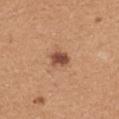Clinical impression: Imaged during a routine full-body skin examination; the lesion was not biopsied and no histopathology is available. Acquisition and patient details: A lesion tile, about 15 mm wide, cut from a 3D total-body photograph. An algorithmic analysis of the crop reported an area of roughly 4.5 mm², an eccentricity of roughly 0.7, and a shape-asymmetry score of about 0.15 (0 = symmetric). The analysis additionally found an average lesion color of about L≈49 a*≈23 b*≈31 (CIELAB), about 14 CIELAB-L* units darker than the surrounding skin, and a normalized border contrast of about 10. And it measured border irregularity of about 1 on a 0–10 scale, a within-lesion color-variation index near 2.5/10, and a peripheral color-asymmetry measure near 0.5. A female subject aged approximately 40. Imaged with white-light lighting. Located on the upper back.